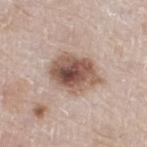Q: Was this lesion biopsied?
A: imaged on a skin check; not biopsied